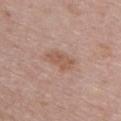follow-up: imaged on a skin check; not biopsied
patient: female, aged around 50
site: the chest
imaging modality: total-body-photography crop, ~15 mm field of view
size: ~4 mm (longest diameter)
illumination: white-light illumination
automated metrics: a footprint of about 6.5 mm² and a shape eccentricity near 0.85; a lesion color around L≈56 a*≈20 b*≈28 in CIELAB, about 8 CIELAB-L* units darker than the surrounding skin, and a lesion-to-skin contrast of about 6.5 (normalized; higher = more distinct); a nevus-likeness score of about 0/100